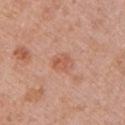Captured during whole-body skin photography for melanoma surveillance; the lesion was not biopsied.
A female patient, approximately 50 years of age.
This image is a 15 mm lesion crop taken from a total-body photograph.
Measured at roughly 2.5 mm in maximum diameter.
Located on the left upper arm.
The tile uses white-light illumination.
Automated image analysis of the tile measured a mean CIELAB color near L≈57 a*≈25 b*≈33, about 8 CIELAB-L* units darker than the surrounding skin, and a normalized border contrast of about 6. The analysis additionally found an automated nevus-likeness rating near 0 out of 100 and a lesion-detection confidence of about 100/100.